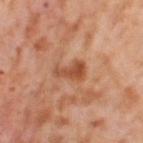{
  "biopsy_status": "not biopsied; imaged during a skin examination",
  "image": {
    "source": "total-body photography crop",
    "field_of_view_mm": 15
  },
  "patient": {
    "sex": "female",
    "age_approx": 55
  },
  "lesion_size": {
    "long_diameter_mm_approx": 3.5
  },
  "site": "right thigh",
  "lighting": "cross-polarized",
  "automated_metrics": {
    "area_mm2_approx": 5.5,
    "eccentricity": 0.85,
    "cielab_L": 51,
    "cielab_a": 26,
    "cielab_b": 36,
    "vs_skin_darker_L": 11.0,
    "vs_skin_contrast_norm": 7.5,
    "border_irregularity_0_10": 5.0,
    "color_variation_0_10": 3.0
  }
}